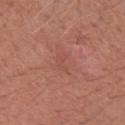Clinical impression:
The lesion was tiled from a total-body skin photograph and was not biopsied.
Image and clinical context:
The lesion-visualizer software estimated a footprint of about 2.5 mm², a shape eccentricity near 0.8, and a symmetry-axis asymmetry near 0.5. The analysis additionally found a mean CIELAB color near L≈50 a*≈26 b*≈28. It also reported a border-irregularity rating of about 5/10 and a peripheral color-asymmetry measure near 0. And it measured a classifier nevus-likeness of about 0/100 and lesion-presence confidence of about 100/100. The recorded lesion diameter is about 2 mm. A region of skin cropped from a whole-body photographic capture, roughly 15 mm wide. The patient is a female about 60 years old. The tile uses white-light illumination. From the left forearm.